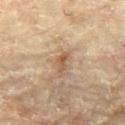notes: imaged on a skin check; not biopsied
acquisition: 15 mm crop, total-body photography
subject: female, aged 78 to 82
lesion size: ~3 mm (longest diameter)
site: the right leg
image-analysis metrics: an area of roughly 3 mm² and a shape-asymmetry score of about 0.4 (0 = symmetric); a mean CIELAB color near L≈50 a*≈16 b*≈30 and about 8 CIELAB-L* units darker than the surrounding skin; border irregularity of about 4 on a 0–10 scale, a within-lesion color-variation index near 2/10, and a peripheral color-asymmetry measure near 0.5; a classifier nevus-likeness of about 30/100 and a detector confidence of about 100 out of 100 that the crop contains a lesion
illumination: cross-polarized illumination